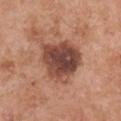imaging modality: total-body-photography crop, ~15 mm field of view | body site: the right upper arm | tile lighting: white-light | lesion diameter: ~5.5 mm (longest diameter) | patient: male, aged around 55.